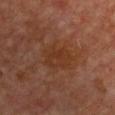Q: Was a biopsy performed?
A: total-body-photography surveillance lesion; no biopsy
Q: What did automated image analysis measure?
A: a lesion area of about 9 mm², an eccentricity of roughly 0.65, and a symmetry-axis asymmetry near 0.15; a lesion color around L≈29 a*≈20 b*≈27 in CIELAB; a border-irregularity rating of about 2/10, internal color variation of about 2.5 on a 0–10 scale, and peripheral color asymmetry of about 1; a nevus-likeness score of about 0/100 and a detector confidence of about 100 out of 100 that the crop contains a lesion
Q: How was this image acquired?
A: ~15 mm crop, total-body skin-cancer survey
Q: Patient demographics?
A: female, roughly 70 years of age
Q: Where on the body is the lesion?
A: the front of the torso